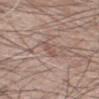biopsy status = total-body-photography surveillance lesion; no biopsy | tile lighting = white-light illumination | image-analysis metrics = roughly 7 lightness units darker than nearby skin and a normalized lesion–skin contrast near 5.5; a border-irregularity index near 4/10 and internal color variation of about 0 on a 0–10 scale; a classifier nevus-likeness of about 0/100 and lesion-presence confidence of about 60/100 | image source = total-body-photography crop, ~15 mm field of view | body site = the mid back | patient = male, aged 58 to 62 | lesion size = about 3 mm.